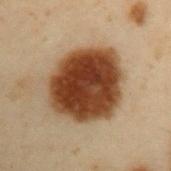acquisition: ~15 mm crop, total-body skin-cancer survey
patient: male, approximately 55 years of age
anatomic site: the left upper arm